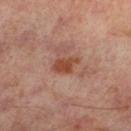biopsy_status: not biopsied; imaged during a skin examination
patient:
  sex: male
  age_approx: 65
lesion_size:
  long_diameter_mm_approx: 3.0
site: left lower leg
automated_metrics:
  cielab_L: 44
  cielab_a: 24
  cielab_b: 30
  vs_skin_darker_L: 10.0
  vs_skin_contrast_norm: 9.0
  nevus_likeness_0_100: 75
image:
  source: total-body photography crop
  field_of_view_mm: 15